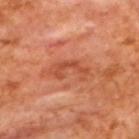| field | value |
|---|---|
| notes | total-body-photography surveillance lesion; no biopsy |
| subject | male, aged approximately 65 |
| size | about 5 mm |
| TBP lesion metrics | an area of roughly 7.5 mm²; an average lesion color of about L≈50 a*≈30 b*≈36 (CIELAB), roughly 8 lightness units darker than nearby skin, and a lesion-to-skin contrast of about 6 (normalized; higher = more distinct); a border-irregularity rating of about 6/10, a color-variation rating of about 2/10, and peripheral color asymmetry of about 1 |
| imaging modality | ~15 mm crop, total-body skin-cancer survey |
| lighting | cross-polarized |